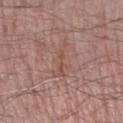<record>
  <biopsy_status>not biopsied; imaged during a skin examination</biopsy_status>
  <site>right forearm</site>
  <image>
    <source>total-body photography crop</source>
    <field_of_view_mm>15</field_of_view_mm>
  </image>
  <patient>
    <sex>male</sex>
    <age_approx>70</age_approx>
  </patient>
</record>